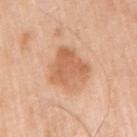Imaged during a routine full-body skin examination; the lesion was not biopsied and no histopathology is available. On the right upper arm. A male subject, about 80 years old. A 15 mm close-up extracted from a 3D total-body photography capture.